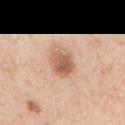• follow-up — catalogued during a skin exam; not biopsied
• illumination — white-light illumination
• lesion diameter — ~3.5 mm (longest diameter)
• image-analysis metrics — a lesion color around L≈60 a*≈22 b*≈32 in CIELAB, roughly 13 lightness units darker than nearby skin, and a normalized border contrast of about 8.5; a border-irregularity rating of about 1.5/10, a color-variation rating of about 6/10, and radial color variation of about 2; lesion-presence confidence of about 100/100
• image — total-body-photography crop, ~15 mm field of view
• site — the right upper arm
• subject — female, aged 48 to 52